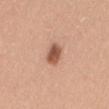Assessment:
Imaged during a routine full-body skin examination; the lesion was not biopsied and no histopathology is available.
Image and clinical context:
Automated image analysis of the tile measured a border-irregularity rating of about 2/10, a color-variation rating of about 3.5/10, and radial color variation of about 1. A female subject, approximately 30 years of age. Captured under white-light illumination. A lesion tile, about 15 mm wide, cut from a 3D total-body photograph. About 3 mm across. On the mid back.